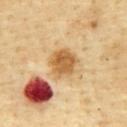Background: The subject is a male aged 63–67. The total-body-photography lesion software estimated a footprint of about 9.5 mm², an eccentricity of roughly 0.25, and two-axis asymmetry of about 0.2. And it measured a color-variation rating of about 3.5/10. A roughly 15 mm field-of-view crop from a total-body skin photograph. Imaged with cross-polarized lighting. Measured at roughly 3.5 mm in maximum diameter. On the front of the torso.